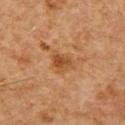biopsy status=no biopsy performed (imaged during a skin exam) | image-analysis metrics=a footprint of about 5.5 mm² and an eccentricity of roughly 0.7; a lesion color around L≈44 a*≈22 b*≈36 in CIELAB and a normalized border contrast of about 7; a classifier nevus-likeness of about 30/100 and lesion-presence confidence of about 100/100 | site=the right upper arm | subject=male, roughly 60 years of age | imaging modality=~15 mm crop, total-body skin-cancer survey.